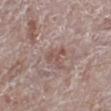Recorded during total-body skin imaging; not selected for excision or biopsy.
The patient is a male aged 68 to 72.
The lesion is on the right leg.
Automated tile analysis of the lesion measured a lesion color around L≈51 a*≈20 b*≈23 in CIELAB, about 8 CIELAB-L* units darker than the surrounding skin, and a lesion-to-skin contrast of about 6.5 (normalized; higher = more distinct).
Approximately 3 mm at its widest.
Cropped from a whole-body photographic skin survey; the tile spans about 15 mm.